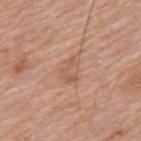Q: Is there a histopathology result?
A: catalogued during a skin exam; not biopsied
Q: Lesion location?
A: the mid back
Q: What are the patient's age and sex?
A: male, about 60 years old
Q: How was the tile lit?
A: white-light
Q: What kind of image is this?
A: total-body-photography crop, ~15 mm field of view
Q: How large is the lesion?
A: ~3.5 mm (longest diameter)